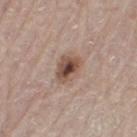workup: catalogued during a skin exam; not biopsied
lesion size: ≈3.5 mm
site: the left thigh
image-analysis metrics: a lesion–skin lightness drop of about 14 and a lesion-to-skin contrast of about 10 (normalized; higher = more distinct); a border-irregularity rating of about 2.5/10, a color-variation rating of about 7.5/10, and radial color variation of about 2; a classifier nevus-likeness of about 90/100 and a detector confidence of about 100 out of 100 that the crop contains a lesion
acquisition: total-body-photography crop, ~15 mm field of view
subject: male, aged 78 to 82